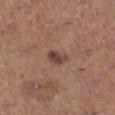biopsy_status: not biopsied; imaged during a skin examination
patient:
  sex: female
  age_approx: 65
image:
  source: total-body photography crop
  field_of_view_mm: 15
site: right lower leg
lighting: white-light
automated_metrics:
  cielab_L: 43
  cielab_a: 19
  cielab_b: 24
  vs_skin_darker_L: 10.0
  vs_skin_contrast_norm: 8.0